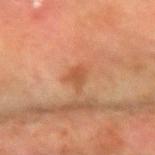This lesion was catalogued during total-body skin photography and was not selected for biopsy.
Longest diameter approximately 3 mm.
Cropped from a whole-body photographic skin survey; the tile spans about 15 mm.
Captured under cross-polarized illumination.
The lesion is on the left forearm.
Automated image analysis of the tile measured a footprint of about 4 mm², a shape eccentricity near 0.75, and two-axis asymmetry of about 0.4. It also reported a mean CIELAB color near L≈47 a*≈23 b*≈34, roughly 8 lightness units darker than nearby skin, and a lesion-to-skin contrast of about 6.5 (normalized; higher = more distinct).
The patient is a female roughly 55 years of age.